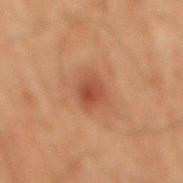  biopsy_status: not biopsied; imaged during a skin examination
  site: mid back
  patient:
    sex: male
    age_approx: 70
  lesion_size:
    long_diameter_mm_approx: 3.0
  automated_metrics:
    area_mm2_approx: 6.0
    eccentricity: 0.6
    shape_asymmetry: 0.2
    cielab_L: 37
    cielab_a: 21
    cielab_b: 27
  image:
    source: total-body photography crop
    field_of_view_mm: 15